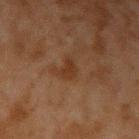notes = imaged on a skin check; not biopsied
subject = male, about 45 years old
anatomic site = the arm
imaging modality = 15 mm crop, total-body photography